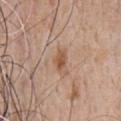Captured during whole-body skin photography for melanoma surveillance; the lesion was not biopsied.
This is a white-light tile.
The patient is a male in their mid- to late 50s.
The recorded lesion diameter is about 2.5 mm.
The lesion is located on the chest.
This image is a 15 mm lesion crop taken from a total-body photograph.